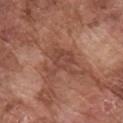Case summary:
• workup · total-body-photography surveillance lesion; no biopsy
• location · the arm
• imaging modality · ~15 mm tile from a whole-body skin photo
• illumination · white-light
• patient · male, aged 73 to 77
• diameter · ≈4.5 mm